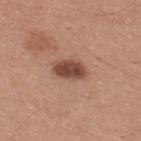location = the upper back | lighting = white-light | subject = male, aged 28–32 | imaging modality = ~15 mm tile from a whole-body skin photo.